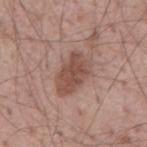The lesion was photographed on a routine skin check and not biopsied; there is no pathology result. This is a white-light tile. The subject is a male aged 53 to 57. A close-up tile cropped from a whole-body skin photograph, about 15 mm across. The lesion-visualizer software estimated internal color variation of about 3 on a 0–10 scale and peripheral color asymmetry of about 1. From the mid back.